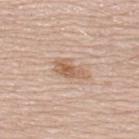Assessment:
No biopsy was performed on this lesion — it was imaged during a full skin examination and was not determined to be concerning.
Background:
The patient is a male in their 70s. A roughly 15 mm field-of-view crop from a total-body skin photograph. On the upper back.